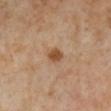biopsy status: catalogued during a skin exam; not biopsied | subject: female, in their 50s | location: the left lower leg | image source: ~15 mm crop, total-body skin-cancer survey.